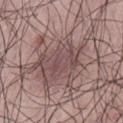workup — imaged on a skin check; not biopsied
site — the left thigh
tile lighting — white-light
subject — male, aged 23 to 27
image source — 15 mm crop, total-body photography
lesion size — ≈9 mm